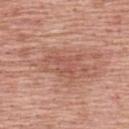The lesion is located on the back. The subject is a male in their 60s. A close-up tile cropped from a whole-body skin photograph, about 15 mm across. The tile uses white-light illumination.